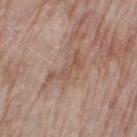biopsy status = no biopsy performed (imaged during a skin exam)
site = the right thigh
acquisition = ~15 mm crop, total-body skin-cancer survey
lighting = white-light illumination
subject = female, aged around 75